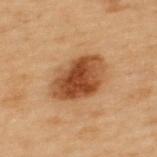{"biopsy_status": "not biopsied; imaged during a skin examination", "lesion_size": {"long_diameter_mm_approx": 6.0}, "patient": {"sex": "male", "age_approx": 55}, "site": "back", "image": {"source": "total-body photography crop", "field_of_view_mm": 15}, "lighting": "cross-polarized"}